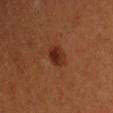<tbp_lesion>
  <automated_metrics>
    <area_mm2_approx>5.0</area_mm2_approx>
    <eccentricity>0.7</eccentricity>
    <shape_asymmetry>0.25</shape_asymmetry>
    <cielab_L>30</cielab_L>
    <cielab_a>25</cielab_a>
    <cielab_b>32</cielab_b>
    <vs_skin_darker_L>9.0</vs_skin_darker_L>
    <vs_skin_contrast_norm>9.0</vs_skin_contrast_norm>
    <border_irregularity_0_10>2.5</border_irregularity_0_10>
    <color_variation_0_10>4.0</color_variation_0_10>
    <peripheral_color_asymmetry>1.5</peripheral_color_asymmetry>
  </automated_metrics>
  <image>
    <source>total-body photography crop</source>
    <field_of_view_mm>15</field_of_view_mm>
  </image>
  <patient>
    <sex>female</sex>
    <age_approx>50</age_approx>
  </patient>
  <lighting>cross-polarized</lighting>
</tbp_lesion>